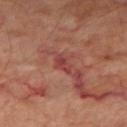Q: Is there a histopathology result?
A: catalogued during a skin exam; not biopsied
Q: How was this image acquired?
A: total-body-photography crop, ~15 mm field of view
Q: What did automated image analysis measure?
A: a lesion color around L≈41 a*≈29 b*≈24 in CIELAB and a normalized lesion–skin contrast near 6.5
Q: How large is the lesion?
A: ≈2.5 mm
Q: Where on the body is the lesion?
A: the right thigh
Q: What lighting was used for the tile?
A: cross-polarized illumination
Q: What are the patient's age and sex?
A: male, approximately 70 years of age